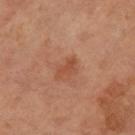The lesion was tiled from a total-body skin photograph and was not biopsied.
A female patient in their mid- to late 60s.
A lesion tile, about 15 mm wide, cut from a 3D total-body photograph.
On the right thigh.
Measured at roughly 2.5 mm in maximum diameter.
This is a cross-polarized tile.